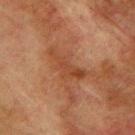Impression: Imaged during a routine full-body skin examination; the lesion was not biopsied and no histopathology is available.